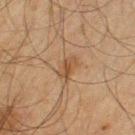notes: imaged on a skin check; not biopsied
anatomic site: the left upper arm
patient: male, aged approximately 65
image: ~15 mm crop, total-body skin-cancer survey
illumination: cross-polarized illumination